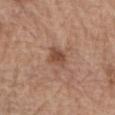Part of a total-body skin-imaging series; this lesion was reviewed on a skin check and was not flagged for biopsy. The lesion is on the right forearm. Automated image analysis of the tile measured a lesion color around L≈48 a*≈21 b*≈29 in CIELAB and a normalized border contrast of about 8. The patient is a male about 70 years old. This is a white-light tile. A roughly 15 mm field-of-view crop from a total-body skin photograph.